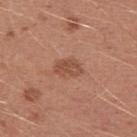Q: Was a biopsy performed?
A: no biopsy performed (imaged during a skin exam)
Q: How large is the lesion?
A: ~3 mm (longest diameter)
Q: How was this image acquired?
A: total-body-photography crop, ~15 mm field of view
Q: Patient demographics?
A: male, aged approximately 25
Q: What is the anatomic site?
A: the arm
Q: How was the tile lit?
A: white-light